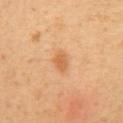Clinical impression: Imaged during a routine full-body skin examination; the lesion was not biopsied and no histopathology is available. Background: On the right upper arm. Approximately 2.5 mm at its widest. A close-up tile cropped from a whole-body skin photograph, about 15 mm across. Captured under cross-polarized illumination. An algorithmic analysis of the crop reported an average lesion color of about L≈61 a*≈24 b*≈41 (CIELAB) and a normalized lesion–skin contrast near 6.5. The analysis additionally found radial color variation of about 0.5. A female patient, about 50 years old.